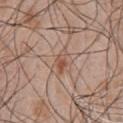Part of a total-body skin-imaging series; this lesion was reviewed on a skin check and was not flagged for biopsy. Longest diameter approximately 3 mm. A 15 mm close-up extracted from a 3D total-body photography capture. The subject is a male aged 48–52. An algorithmic analysis of the crop reported a normalized lesion–skin contrast near 7. The lesion is on the chest. The tile uses white-light illumination.